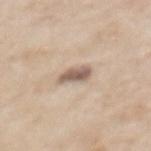The lesion was photographed on a routine skin check and not biopsied; there is no pathology result. The subject is a female roughly 60 years of age. Located on the upper back. A 15 mm crop from a total-body photograph taken for skin-cancer surveillance. Automated image analysis of the tile measured an eccentricity of roughly 0.8 and two-axis asymmetry of about 0.25. The software also gave a border-irregularity index near 2.5/10, internal color variation of about 3 on a 0–10 scale, and radial color variation of about 1. The software also gave a detector confidence of about 90 out of 100 that the crop contains a lesion. Captured under white-light illumination. Approximately 3 mm at its widest.